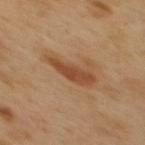biopsy status: imaged on a skin check; not biopsied
acquisition: ~15 mm crop, total-body skin-cancer survey
body site: the mid back
patient: male, aged 48 to 52
lighting: cross-polarized illumination